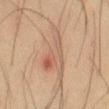Notes:
* follow-up — catalogued during a skin exam; not biopsied
* body site — the right thigh
* subject — male, aged 33–37
* tile lighting — cross-polarized illumination
* automated metrics — a footprint of about 15 mm² and a shape-asymmetry score of about 0.6 (0 = symmetric)
* acquisition — total-body-photography crop, ~15 mm field of view
* diameter — about 8.5 mm